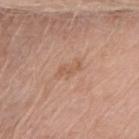This lesion was catalogued during total-body skin photography and was not selected for biopsy. From the left upper arm. A female patient roughly 75 years of age. An algorithmic analysis of the crop reported a footprint of about 4.5 mm², a shape eccentricity near 0.9, and two-axis asymmetry of about 0.4. It also reported roughly 6 lightness units darker than nearby skin and a normalized lesion–skin contrast near 5. The software also gave a detector confidence of about 100 out of 100 that the crop contains a lesion. A close-up tile cropped from a whole-body skin photograph, about 15 mm across. About 3.5 mm across. Captured under white-light illumination.